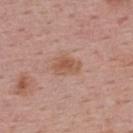Clinical impression: Imaged during a routine full-body skin examination; the lesion was not biopsied and no histopathology is available. Background: A female patient, roughly 55 years of age. The lesion is on the upper back. This image is a 15 mm lesion crop taken from a total-body photograph. The recorded lesion diameter is about 3.5 mm. This is a white-light tile.